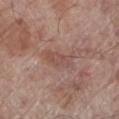– notes · catalogued during a skin exam; not biopsied
– body site · the left lower leg
– image · ~15 mm tile from a whole-body skin photo
– subject · male, aged approximately 70